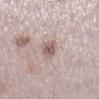workup: catalogued during a skin exam; not biopsied | anatomic site: the right lower leg | image source: ~15 mm crop, total-body skin-cancer survey | patient: female, aged 28 to 32.